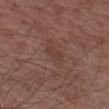Assessment: Part of a total-body skin-imaging series; this lesion was reviewed on a skin check and was not flagged for biopsy. Context: About 3 mm across. Cropped from a whole-body photographic skin survey; the tile spans about 15 mm. A male subject, in their mid-60s. The lesion-visualizer software estimated an outline eccentricity of about 0.85 (0 = round, 1 = elongated) and two-axis asymmetry of about 0.35. It also reported an average lesion color of about L≈39 a*≈20 b*≈25 (CIELAB), about 5 CIELAB-L* units darker than the surrounding skin, and a normalized border contrast of about 4.5. It also reported a border-irregularity index near 3.5/10, a color-variation rating of about 1/10, and peripheral color asymmetry of about 0. It also reported a lesion-detection confidence of about 100/100. The lesion is located on the left forearm. Imaged with white-light lighting.